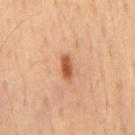• notes: no biopsy performed (imaged during a skin exam)
• body site: the front of the torso
• subject: male, roughly 60 years of age
• lesion diameter: ~3 mm (longest diameter)
• tile lighting: cross-polarized illumination
• image source: ~15 mm crop, total-body skin-cancer survey